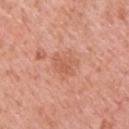location: the upper back | acquisition: ~15 mm tile from a whole-body skin photo | subject: male, aged 58 to 62.